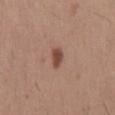Case summary:
* follow-up · catalogued during a skin exam; not biopsied
* location · the mid back
* lighting · white-light
* automated metrics · an area of roughly 4 mm² and two-axis asymmetry of about 0.3; a mean CIELAB color near L≈47 a*≈20 b*≈27; border irregularity of about 2.5 on a 0–10 scale
* acquisition · ~15 mm crop, total-body skin-cancer survey
* patient · male, roughly 65 years of age